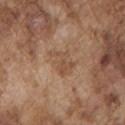Q: Was this lesion biopsied?
A: no biopsy performed (imaged during a skin exam)
Q: What lighting was used for the tile?
A: white-light
Q: What is the lesion's diameter?
A: about 3.5 mm
Q: Where on the body is the lesion?
A: the arm
Q: How was this image acquired?
A: total-body-photography crop, ~15 mm field of view
Q: What are the patient's age and sex?
A: male, roughly 75 years of age